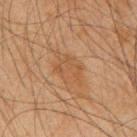Clinical impression:
Recorded during total-body skin imaging; not selected for excision or biopsy.
Context:
An algorithmic analysis of the crop reported an outline eccentricity of about 0.75 (0 = round, 1 = elongated). The software also gave an automated nevus-likeness rating near 0 out of 100 and lesion-presence confidence of about 100/100. A male patient aged 63–67. A region of skin cropped from a whole-body photographic capture, roughly 15 mm wide. The lesion is located on the upper back. This is a cross-polarized tile. Measured at roughly 5 mm in maximum diameter.